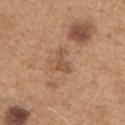Recorded during total-body skin imaging; not selected for excision or biopsy. Imaged with white-light lighting. A close-up tile cropped from a whole-body skin photograph, about 15 mm across. The lesion is located on the chest. The subject is a male about 65 years old.